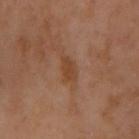- notes · no biopsy performed (imaged during a skin exam)
- site · the right upper arm
- automated metrics · a normalized border contrast of about 6.5
- tile lighting · cross-polarized
- image source · 15 mm crop, total-body photography
- diameter · ≈3 mm
- patient · female, about 55 years old